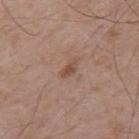biopsy status = total-body-photography surveillance lesion; no biopsy | subject = male, aged 68–72 | imaging modality = 15 mm crop, total-body photography | lesion diameter = ~2.5 mm (longest diameter) | anatomic site = the back | lighting = white-light illumination | automated lesion analysis = an automated nevus-likeness rating near 5 out of 100.